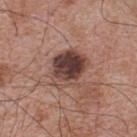follow-up = no biopsy performed (imaged during a skin exam)
patient = male, aged approximately 65
site = the upper back
imaging modality = ~15 mm tile from a whole-body skin photo